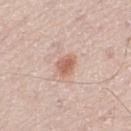Captured during whole-body skin photography for melanoma surveillance; the lesion was not biopsied. The patient is a male about 70 years old. From the left thigh. About 2.5 mm across. Cropped from a total-body skin-imaging series; the visible field is about 15 mm.